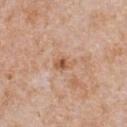Captured during whole-body skin photography for melanoma surveillance; the lesion was not biopsied. Captured under white-light illumination. The recorded lesion diameter is about 2.5 mm. Automated tile analysis of the lesion measured a mean CIELAB color near L≈58 a*≈21 b*≈33 and about 9 CIELAB-L* units darker than the surrounding skin. The software also gave a border-irregularity rating of about 3.5/10, a within-lesion color-variation index near 4/10, and a peripheral color-asymmetry measure near 1.5. On the chest. A close-up tile cropped from a whole-body skin photograph, about 15 mm across. A male patient, aged 63–67.